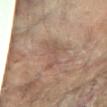Q: Was this lesion biopsied?
A: catalogued during a skin exam; not biopsied
Q: What kind of image is this?
A: total-body-photography crop, ~15 mm field of view
Q: Lesion location?
A: the left arm
Q: Who is the patient?
A: female, about 80 years old